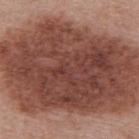Assessment:
The lesion was photographed on a routine skin check and not biopsied; there is no pathology result.
Image and clinical context:
The tile uses white-light illumination. A region of skin cropped from a whole-body photographic capture, roughly 15 mm wide. A female subject aged 48–52. Automated image analysis of the tile measured an average lesion color of about L≈42 a*≈23 b*≈25 (CIELAB), a lesion–skin lightness drop of about 15, and a normalized border contrast of about 11. The analysis additionally found a border-irregularity index near 6.5/10, a within-lesion color-variation index near 4.5/10, and peripheral color asymmetry of about 1.5. On the upper back.